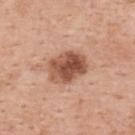The lesion was photographed on a routine skin check and not biopsied; there is no pathology result.
A 15 mm close-up extracted from a 3D total-body photography capture.
The total-body-photography lesion software estimated an outline eccentricity of about 0.7 (0 = round, 1 = elongated) and a symmetry-axis asymmetry near 0.15. It also reported an average lesion color of about L≈53 a*≈23 b*≈31 (CIELAB). The analysis additionally found a border-irregularity rating of about 1.5/10, a color-variation rating of about 7.5/10, and peripheral color asymmetry of about 2.5. It also reported a nevus-likeness score of about 70/100 and lesion-presence confidence of about 100/100.
Located on the upper back.
A female subject, about 50 years old.